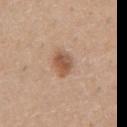| key | value |
|---|---|
| workup | catalogued during a skin exam; not biopsied |
| location | the chest |
| image source | 15 mm crop, total-body photography |
| tile lighting | white-light illumination |
| TBP lesion metrics | a footprint of about 5.5 mm², a shape eccentricity near 0.75, and a shape-asymmetry score of about 0.25 (0 = symmetric); a lesion color around L≈54 a*≈20 b*≈31 in CIELAB and a lesion–skin lightness drop of about 12; a border-irregularity rating of about 2/10, internal color variation of about 4.5 on a 0–10 scale, and radial color variation of about 1.5 |
| lesion size | about 3 mm |
| subject | male, aged approximately 40 |